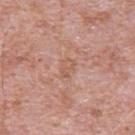This lesion was catalogued during total-body skin photography and was not selected for biopsy. Cropped from a whole-body photographic skin survey; the tile spans about 15 mm. Longest diameter approximately 2.5 mm. A male patient, in their 50s. On the upper back. Captured under white-light illumination.